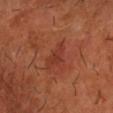{"biopsy_status": "not biopsied; imaged during a skin examination", "lesion_size": {"long_diameter_mm_approx": 3.0}, "site": "right forearm", "automated_metrics": {"area_mm2_approx": 4.0, "eccentricity": 0.85, "shape_asymmetry": 0.45, "cielab_L": 34, "cielab_a": 28, "cielab_b": 29, "vs_skin_darker_L": 6.0, "nevus_likeness_0_100": 55, "lesion_detection_confidence_0_100": 100}, "lighting": "cross-polarized", "image": {"source": "total-body photography crop", "field_of_view_mm": 15}, "patient": {"sex": "male", "age_approx": 60}}